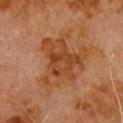Q: Was this lesion biopsied?
A: catalogued during a skin exam; not biopsied
Q: What lighting was used for the tile?
A: cross-polarized
Q: Patient demographics?
A: male, aged around 80
Q: What is the anatomic site?
A: the upper back
Q: Lesion size?
A: ~7 mm (longest diameter)
Q: What is the imaging modality?
A: total-body-photography crop, ~15 mm field of view